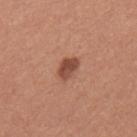| feature | finding |
|---|---|
| biopsy status | imaged on a skin check; not biopsied |
| lighting | white-light |
| body site | the upper back |
| size | about 3 mm |
| patient | female, roughly 50 years of age |
| acquisition | total-body-photography crop, ~15 mm field of view |